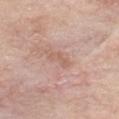Acquisition and patient details: Captured under white-light illumination. Longest diameter approximately 2.5 mm. A close-up tile cropped from a whole-body skin photograph, about 15 mm across. Automated image analysis of the tile measured a mean CIELAB color near L≈60 a*≈19 b*≈28, roughly 6 lightness units darker than nearby skin, and a normalized border contrast of about 5. And it measured border irregularity of about 6 on a 0–10 scale, a color-variation rating of about 0/10, and a peripheral color-asymmetry measure near 0. The software also gave a nevus-likeness score of about 0/100 and a lesion-detection confidence of about 100/100. The lesion is located on the chest. The patient is a female about 70 years old.